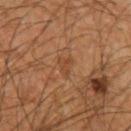{
  "biopsy_status": "not biopsied; imaged during a skin examination",
  "lesion_size": {
    "long_diameter_mm_approx": 2.5
  },
  "site": "left upper arm",
  "image": {
    "source": "total-body photography crop",
    "field_of_view_mm": 15
  },
  "patient": {
    "sex": "male",
    "age_approx": 65
  }
}